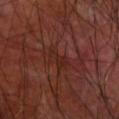notes = catalogued during a skin exam; not biopsied | lighting = cross-polarized illumination | subject = male, approximately 60 years of age | location = the right upper arm | image source = ~15 mm crop, total-body skin-cancer survey | size = ~3.5 mm (longest diameter).